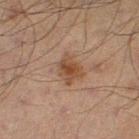The lesion was photographed on a routine skin check and not biopsied; there is no pathology result. From the leg. A male patient, aged 68–72. The lesion's longest dimension is about 3.5 mm. This is a cross-polarized tile. A 15 mm close-up extracted from a 3D total-body photography capture.